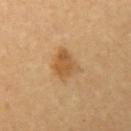Impression: The lesion was tiled from a total-body skin photograph and was not biopsied. Context: A close-up tile cropped from a whole-body skin photograph, about 15 mm across. From the left upper arm. Imaged with cross-polarized lighting. A female subject, approximately 60 years of age. The total-body-photography lesion software estimated border irregularity of about 3 on a 0–10 scale and radial color variation of about 1. It also reported a classifier nevus-likeness of about 80/100 and a detector confidence of about 100 out of 100 that the crop contains a lesion. The recorded lesion diameter is about 3 mm.